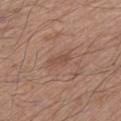<record>
  <biopsy_status>not biopsied; imaged during a skin examination</biopsy_status>
  <site>left lower leg</site>
  <patient>
    <sex>male</sex>
    <age_approx>65</age_approx>
  </patient>
  <lesion_size>
    <long_diameter_mm_approx>2.5</long_diameter_mm_approx>
  </lesion_size>
  <automated_metrics>
    <nevus_likeness_0_100>0</nevus_likeness_0_100>
  </automated_metrics>
  <image>
    <source>total-body photography crop</source>
    <field_of_view_mm>15</field_of_view_mm>
  </image>
</record>